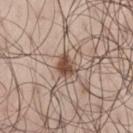biopsy status — total-body-photography surveillance lesion; no biopsy
image source — ~15 mm tile from a whole-body skin photo
subject — male, aged 43 to 47
lesion size — about 2.5 mm
automated lesion analysis — a footprint of about 5 mm², an outline eccentricity of about 0.55 (0 = round, 1 = elongated), and a symmetry-axis asymmetry near 0.3; a within-lesion color-variation index near 3.5/10 and peripheral color asymmetry of about 1; a lesion-detection confidence of about 100/100
anatomic site — the leg